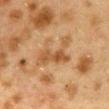Part of a total-body skin-imaging series; this lesion was reviewed on a skin check and was not flagged for biopsy.
Captured under cross-polarized illumination.
A region of skin cropped from a whole-body photographic capture, roughly 15 mm wide.
The lesion's longest dimension is about 5.5 mm.
The lesion is located on the mid back.
A female patient, about 40 years old.
The lesion-visualizer software estimated a lesion area of about 10 mm², a shape eccentricity near 0.85, and a symmetry-axis asymmetry near 0.55. The software also gave a mean CIELAB color near L≈45 a*≈18 b*≈34, about 8 CIELAB-L* units darker than the surrounding skin, and a normalized lesion–skin contrast near 6.5. It also reported a border-irregularity rating of about 7.5/10, a color-variation rating of about 3.5/10, and peripheral color asymmetry of about 1. The analysis additionally found a nevus-likeness score of about 0/100 and a lesion-detection confidence of about 80/100.